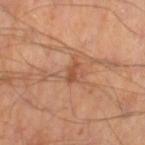Findings:
– notes: imaged on a skin check; not biopsied
– image: 15 mm crop, total-body photography
– TBP lesion metrics: a mean CIELAB color near L≈49 a*≈24 b*≈34, roughly 9 lightness units darker than nearby skin, and a lesion-to-skin contrast of about 6.5 (normalized; higher = more distinct)
– anatomic site: the left thigh
– subject: male, aged 43–47
– illumination: cross-polarized illumination